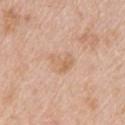Assessment:
This lesion was catalogued during total-body skin photography and was not selected for biopsy.
Acquisition and patient details:
On the upper back. Imaged with white-light lighting. An algorithmic analysis of the crop reported a color-variation rating of about 4/10 and radial color variation of about 1.5. A 15 mm close-up tile from a total-body photography series done for melanoma screening. A female subject aged approximately 45. Approximately 3 mm at its widest.